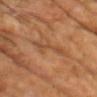Q: Was this lesion biopsied?
A: no biopsy performed (imaged during a skin exam)
Q: How was this image acquired?
A: ~15 mm tile from a whole-body skin photo
Q: How large is the lesion?
A: ~4.5 mm (longest diameter)
Q: Who is the patient?
A: male, roughly 70 years of age
Q: What is the anatomic site?
A: the chest